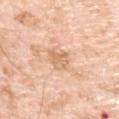Recorded during total-body skin imaging; not selected for excision or biopsy. A 15 mm crop from a total-body photograph taken for skin-cancer surveillance. Captured under white-light illumination. Automated tile analysis of the lesion measured a footprint of about 5 mm², an eccentricity of roughly 0.3, and two-axis asymmetry of about 0.35. It also reported radial color variation of about 1. And it measured a nevus-likeness score of about 0/100 and lesion-presence confidence of about 100/100. The recorded lesion diameter is about 2.5 mm. The patient is a male roughly 70 years of age. From the chest.